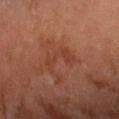Findings:
* notes — no biopsy performed (imaged during a skin exam)
* subject — male, about 65 years old
* image — ~15 mm tile from a whole-body skin photo